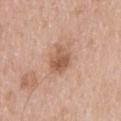Impression: Part of a total-body skin-imaging series; this lesion was reviewed on a skin check and was not flagged for biopsy. Context: The total-body-photography lesion software estimated an average lesion color of about L≈56 a*≈21 b*≈30 (CIELAB), roughly 11 lightness units darker than nearby skin, and a lesion-to-skin contrast of about 7.5 (normalized; higher = more distinct). And it measured a within-lesion color-variation index near 4.5/10 and a peripheral color-asymmetry measure near 2. It also reported a nevus-likeness score of about 10/100 and a detector confidence of about 100 out of 100 that the crop contains a lesion. On the upper back. Longest diameter approximately 3.5 mm. The patient is a male roughly 40 years of age. A roughly 15 mm field-of-view crop from a total-body skin photograph. Captured under white-light illumination.